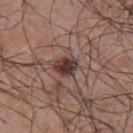workup: total-body-photography surveillance lesion; no biopsy | anatomic site: the back | patient: male, aged 48 to 52 | size: ~3 mm (longest diameter) | imaging modality: total-body-photography crop, ~15 mm field of view.